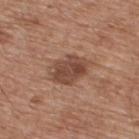Captured during whole-body skin photography for melanoma surveillance; the lesion was not biopsied. An algorithmic analysis of the crop reported a color-variation rating of about 4/10 and a peripheral color-asymmetry measure near 1.5. The lesion is on the upper back. A 15 mm crop from a total-body photograph taken for skin-cancer surveillance. The subject is a male approximately 65 years of age. Approximately 4.5 mm at its widest.